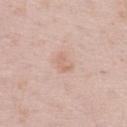• biopsy status — imaged on a skin check; not biopsied
• automated metrics — a lesion area of about 4.5 mm² and an eccentricity of roughly 0.7; roughly 6 lightness units darker than nearby skin and a normalized lesion–skin contrast near 4.5; a nevus-likeness score of about 10/100 and a lesion-detection confidence of about 100/100
• imaging modality — ~15 mm tile from a whole-body skin photo
• lesion size — about 2.5 mm
• tile lighting — white-light illumination
• subject — male, about 60 years old
• site — the left thigh